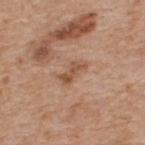The lesion was tiled from a total-body skin photograph and was not biopsied.
On the upper back.
A male patient, aged 58–62.
This is a white-light tile.
The lesion-visualizer software estimated an eccentricity of roughly 0.9. It also reported a lesion–skin lightness drop of about 9 and a normalized lesion–skin contrast near 7. It also reported a border-irregularity rating of about 4.5/10, a within-lesion color-variation index near 3/10, and a peripheral color-asymmetry measure near 1.
This image is a 15 mm lesion crop taken from a total-body photograph.